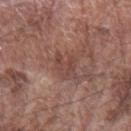The lesion was tiled from a total-body skin photograph and was not biopsied. Cropped from a whole-body photographic skin survey; the tile spans about 15 mm. On the arm. A male subject, approximately 75 years of age.